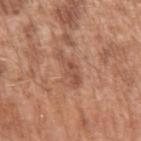The lesion was photographed on a routine skin check and not biopsied; there is no pathology result. The lesion is located on the left upper arm. Approximately 4.5 mm at its widest. A 15 mm close-up tile from a total-body photography series done for melanoma screening. The patient is a male aged 63 to 67. The total-body-photography lesion software estimated a classifier nevus-likeness of about 0/100 and lesion-presence confidence of about 80/100.